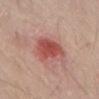notes — imaged on a skin check; not biopsied | image source — ~15 mm crop, total-body skin-cancer survey | patient — male, about 40 years old | body site — the back | automated lesion analysis — a nevus-likeness score of about 80/100 and a detector confidence of about 100 out of 100 that the crop contains a lesion | lighting — white-light.